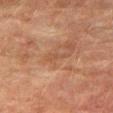Assessment: Recorded during total-body skin imaging; not selected for excision or biopsy. Context: A male patient about 75 years old. Longest diameter approximately 3.5 mm. The lesion is located on the right thigh. An algorithmic analysis of the crop reported an area of roughly 2.5 mm² and an eccentricity of roughly 0.95. The analysis additionally found border irregularity of about 5.5 on a 0–10 scale, a color-variation rating of about 0/10, and a peripheral color-asymmetry measure near 0. The analysis additionally found a nevus-likeness score of about 0/100 and a detector confidence of about 100 out of 100 that the crop contains a lesion. A 15 mm crop from a total-body photograph taken for skin-cancer surveillance.